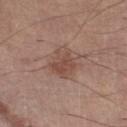workup: imaged on a skin check; not biopsied
image: ~15 mm crop, total-body skin-cancer survey
patient: male, approximately 60 years of age
TBP lesion metrics: an outline eccentricity of about 0.6 (0 = round, 1 = elongated); an average lesion color of about L≈48 a*≈19 b*≈25 (CIELAB), about 8 CIELAB-L* units darker than the surrounding skin, and a lesion-to-skin contrast of about 6.5 (normalized; higher = more distinct)
size: ≈4 mm
tile lighting: white-light illumination
site: the right lower leg